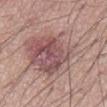biopsy status=total-body-photography surveillance lesion; no biopsy | illumination=white-light | location=the abdomen | diameter=about 12 mm | subject=male, aged 53 to 57 | imaging modality=15 mm crop, total-body photography.